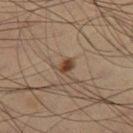Acquisition and patient details: The subject is a male aged approximately 65. A lesion tile, about 15 mm wide, cut from a 3D total-body photograph. The lesion is on the leg. The tile uses cross-polarized illumination.